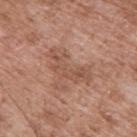On the upper back.
Longest diameter approximately 5.5 mm.
A roughly 15 mm field-of-view crop from a total-body skin photograph.
A male patient, approximately 55 years of age.
The lesion-visualizer software estimated a lesion area of about 9 mm² and two-axis asymmetry of about 0.6. The analysis additionally found about 8 CIELAB-L* units darker than the surrounding skin and a normalized border contrast of about 5.5. And it measured border irregularity of about 8 on a 0–10 scale and radial color variation of about 0.5.